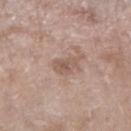workup: no biopsy performed (imaged during a skin exam) | size: ~3 mm (longest diameter) | subject: female, aged approximately 75 | tile lighting: white-light illumination | image: 15 mm crop, total-body photography | location: the right lower leg | automated lesion analysis: a lesion area of about 4.5 mm², an eccentricity of roughly 0.75, and a shape-asymmetry score of about 0.35 (0 = symmetric); border irregularity of about 3 on a 0–10 scale, a color-variation rating of about 2.5/10, and peripheral color asymmetry of about 1; an automated nevus-likeness rating near 5 out of 100 and a lesion-detection confidence of about 100/100.